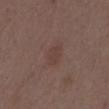patient: male, aged around 50
location: the chest
imaging modality: ~15 mm tile from a whole-body skin photo